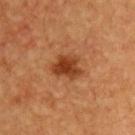{"biopsy_status": "not biopsied; imaged during a skin examination", "patient": {"sex": "male", "age_approx": 60}, "lighting": "cross-polarized", "site": "chest", "lesion_size": {"long_diameter_mm_approx": 3.5}, "image": {"source": "total-body photography crop", "field_of_view_mm": 15}}